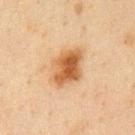| field | value |
|---|---|
| workup | total-body-photography surveillance lesion; no biopsy |
| imaging modality | total-body-photography crop, ~15 mm field of view |
| automated lesion analysis | peripheral color asymmetry of about 2 |
| lesion size | ~4.5 mm (longest diameter) |
| site | the front of the torso |
| subject | male, aged approximately 60 |